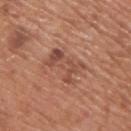Q: Was this lesion biopsied?
A: catalogued during a skin exam; not biopsied
Q: What kind of image is this?
A: ~15 mm tile from a whole-body skin photo
Q: What is the lesion's diameter?
A: ~5 mm (longest diameter)
Q: Lesion location?
A: the upper back
Q: Illumination type?
A: white-light
Q: Who is the patient?
A: male, roughly 65 years of age
Q: Automated lesion metrics?
A: a footprint of about 9 mm² and two-axis asymmetry of about 0.35; a border-irregularity index near 7/10, a color-variation rating of about 4.5/10, and radial color variation of about 1